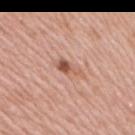follow-up=no biopsy performed (imaged during a skin exam)
patient=female, roughly 40 years of age
anatomic site=the right upper arm
imaging modality=~15 mm tile from a whole-body skin photo